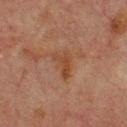Captured during whole-body skin photography for melanoma surveillance; the lesion was not biopsied. Imaged with cross-polarized lighting. A male subject aged 63–67. A close-up tile cropped from a whole-body skin photograph, about 15 mm across. The lesion is located on the upper back. The lesion's longest dimension is about 3.5 mm. An algorithmic analysis of the crop reported an area of roughly 4.5 mm², an eccentricity of roughly 0.8, and a shape-asymmetry score of about 0.55 (0 = symmetric). It also reported about 6 CIELAB-L* units darker than the surrounding skin. The analysis additionally found a color-variation rating of about 1.5/10 and a peripheral color-asymmetry measure near 0.5.